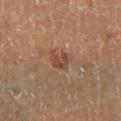{"biopsy_status": "not biopsied; imaged during a skin examination", "patient": {"sex": "male", "age_approx": 60}, "image": {"source": "total-body photography crop", "field_of_view_mm": 15}, "site": "right lower leg", "lesion_size": {"long_diameter_mm_approx": 2.5}, "lighting": "cross-polarized"}